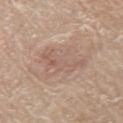No biopsy was performed on this lesion — it was imaged during a full skin examination and was not determined to be concerning.
A male subject aged 78 to 82.
Captured under white-light illumination.
On the left upper arm.
About 6 mm across.
A 15 mm close-up extracted from a 3D total-body photography capture.